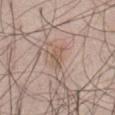{
  "biopsy_status": "not biopsied; imaged during a skin examination",
  "patient": {
    "sex": "male",
    "age_approx": 50
  },
  "site": "abdomen",
  "image": {
    "source": "total-body photography crop",
    "field_of_view_mm": 15
  }
}